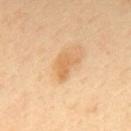Notes:
* notes: catalogued during a skin exam; not biopsied
* image: 15 mm crop, total-body photography
* patient: male, aged around 60
* site: the mid back